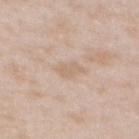Impression: The lesion was photographed on a routine skin check and not biopsied; there is no pathology result.